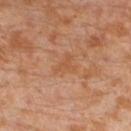{
  "lighting": "cross-polarized",
  "patient": {
    "sex": "male",
    "age_approx": 30
  },
  "image": {
    "source": "total-body photography crop",
    "field_of_view_mm": 15
  },
  "site": "left lower leg",
  "automated_metrics": {
    "area_mm2_approx": 5.0,
    "shape_asymmetry": 0.5
  }
}